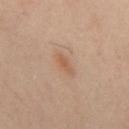Q: What is the lesion's diameter?
A: ≈3 mm
Q: What kind of image is this?
A: 15 mm crop, total-body photography
Q: What are the patient's age and sex?
A: male, aged 38–42
Q: Where on the body is the lesion?
A: the back